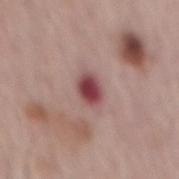Recorded during total-body skin imaging; not selected for excision or biopsy. A roughly 15 mm field-of-view crop from a total-body skin photograph. A male patient aged approximately 65. The lesion is on the mid back. Captured under white-light illumination. About 3.5 mm across.